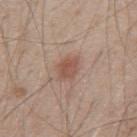Clinical impression:
Part of a total-body skin-imaging series; this lesion was reviewed on a skin check and was not flagged for biopsy.
Background:
A 15 mm crop from a total-body photograph taken for skin-cancer surveillance. The patient is a male roughly 50 years of age. On the mid back.